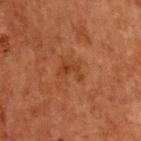biopsy status — no biopsy performed (imaged during a skin exam)
acquisition — ~15 mm crop, total-body skin-cancer survey
size — about 3 mm
image-analysis metrics — an eccentricity of roughly 0.8 and a shape-asymmetry score of about 0.55 (0 = symmetric); roughly 6 lightness units darker than nearby skin and a lesion-to-skin contrast of about 6 (normalized; higher = more distinct)
location — the chest
subject — male, aged around 65